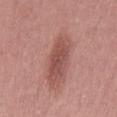Q: Is there a histopathology result?
A: no biopsy performed (imaged during a skin exam)
Q: What is the lesion's diameter?
A: ~5.5 mm (longest diameter)
Q: Where on the body is the lesion?
A: the left thigh
Q: What are the patient's age and sex?
A: female, aged around 40
Q: How was this image acquired?
A: total-body-photography crop, ~15 mm field of view
Q: What did automated image analysis measure?
A: a lesion color around L≈49 a*≈25 b*≈23 in CIELAB, roughly 11 lightness units darker than nearby skin, and a normalized border contrast of about 8; a within-lesion color-variation index near 2.5/10 and peripheral color asymmetry of about 1
Q: How was the tile lit?
A: white-light illumination